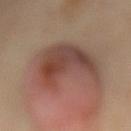Captured during whole-body skin photography for melanoma surveillance; the lesion was not biopsied.
Imaged with cross-polarized lighting.
From the mid back.
A female patient about 55 years old.
Approximately 8.5 mm at its widest.
Cropped from a total-body skin-imaging series; the visible field is about 15 mm.